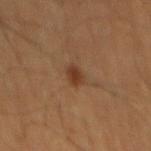Q: Was this lesion biopsied?
A: imaged on a skin check; not biopsied
Q: What is the imaging modality?
A: ~15 mm crop, total-body skin-cancer survey
Q: Lesion location?
A: the mid back
Q: Lesion size?
A: ≈2.5 mm
Q: How was the tile lit?
A: cross-polarized illumination
Q: Patient demographics?
A: male, aged 58 to 62